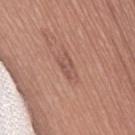notes: no biopsy performed (imaged during a skin exam)
acquisition: 15 mm crop, total-body photography
patient: female, aged approximately 75
illumination: white-light
location: the right thigh
diameter: ≈3 mm
automated metrics: a lesion area of about 4 mm², an eccentricity of roughly 0.9, and a shape-asymmetry score of about 0.4 (0 = symmetric); an average lesion color of about L≈53 a*≈21 b*≈26 (CIELAB) and roughly 7 lightness units darker than nearby skin; border irregularity of about 4 on a 0–10 scale and internal color variation of about 2.5 on a 0–10 scale